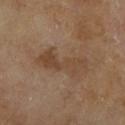<record>
  <biopsy_status>not biopsied; imaged during a skin examination</biopsy_status>
  <patient>
    <sex>male</sex>
    <age_approx>65</age_approx>
  </patient>
  <lighting>cross-polarized</lighting>
  <automated_metrics>
    <cielab_L>43</cielab_L>
    <cielab_a>16</cielab_a>
    <cielab_b>29</cielab_b>
    <vs_skin_darker_L>7.0</vs_skin_darker_L>
  </automated_metrics>
  <site>right lower leg</site>
  <lesion_size>
    <long_diameter_mm_approx>6.5</long_diameter_mm_approx>
  </lesion_size>
  <image>
    <source>total-body photography crop</source>
    <field_of_view_mm>15</field_of_view_mm>
  </image>
</record>